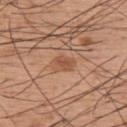workup: no biopsy performed (imaged during a skin exam)
image: ~15 mm crop, total-body skin-cancer survey
location: the back
patient: male, in their mid- to late 50s